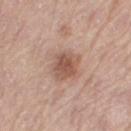Clinical impression:
Recorded during total-body skin imaging; not selected for excision or biopsy.
Acquisition and patient details:
Measured at roughly 3.5 mm in maximum diameter. The lesion is on the left thigh. A female subject, aged 63 to 67. Imaged with white-light lighting. A lesion tile, about 15 mm wide, cut from a 3D total-body photograph.